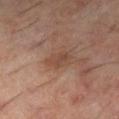| field | value |
|---|---|
| biopsy status | no biopsy performed (imaged during a skin exam) |
| lighting | cross-polarized illumination |
| site | the right thigh |
| subject | male, aged around 60 |
| size | ≈3 mm |
| image source | ~15 mm tile from a whole-body skin photo |